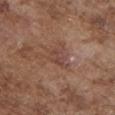follow-up = total-body-photography surveillance lesion; no biopsy | subject = male, in their mid- to late 70s | image source = total-body-photography crop, ~15 mm field of view | anatomic site = the chest | image-analysis metrics = a footprint of about 4.5 mm², a shape eccentricity near 0.7, and two-axis asymmetry of about 0.3; about 6 CIELAB-L* units darker than the surrounding skin and a normalized border contrast of about 5.5; a classifier nevus-likeness of about 0/100 | tile lighting = white-light | diameter = ≈3 mm.